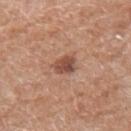Assessment:
Part of a total-body skin-imaging series; this lesion was reviewed on a skin check and was not flagged for biopsy.
Image and clinical context:
The lesion is on the right forearm. Longest diameter approximately 2.5 mm. A close-up tile cropped from a whole-body skin photograph, about 15 mm across. The patient is a female approximately 75 years of age. The lesion-visualizer software estimated an area of roughly 4.5 mm², an eccentricity of roughly 0.65, and two-axis asymmetry of about 0.25. This is a white-light tile.